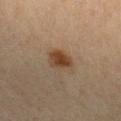Impression:
Imaged during a routine full-body skin examination; the lesion was not biopsied and no histopathology is available.
Clinical summary:
A 15 mm close-up tile from a total-body photography series done for melanoma screening. A female patient, in their mid-40s. The lesion is on the arm. An algorithmic analysis of the crop reported an average lesion color of about L≈36 a*≈16 b*≈27 (CIELAB) and roughly 10 lightness units darker than nearby skin. And it measured lesion-presence confidence of about 100/100. Captured under cross-polarized illumination.